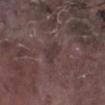Impression:
Captured during whole-body skin photography for melanoma surveillance; the lesion was not biopsied.
Background:
The lesion's longest dimension is about 2.5 mm. A 15 mm close-up tile from a total-body photography series done for melanoma screening. The lesion-visualizer software estimated a lesion area of about 4.5 mm², an eccentricity of roughly 0.7, and two-axis asymmetry of about 0.3. And it measured a lesion color around L≈34 a*≈14 b*≈14 in CIELAB, roughly 6 lightness units darker than nearby skin, and a normalized lesion–skin contrast near 5.5. The patient is a male about 75 years old. On the left lower leg. Imaged with white-light lighting.